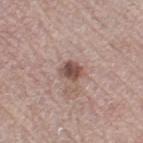notes: imaged on a skin check; not biopsied
subject: female, aged around 65
image: ~15 mm crop, total-body skin-cancer survey
illumination: white-light
automated lesion analysis: a lesion area of about 4.5 mm², an outline eccentricity of about 0.45 (0 = round, 1 = elongated), and a symmetry-axis asymmetry near 0.15; an automated nevus-likeness rating near 80 out of 100
lesion diameter: ≈2.5 mm
body site: the right thigh